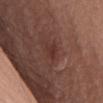Notes:
– follow-up · no biopsy performed (imaged during a skin exam)
– automated lesion analysis · an area of roughly 3.5 mm² and two-axis asymmetry of about 0.35; an average lesion color of about L≈33 a*≈22 b*≈23 (CIELAB), roughly 6 lightness units darker than nearby skin, and a lesion-to-skin contrast of about 6 (normalized; higher = more distinct); an automated nevus-likeness rating near 15 out of 100
– anatomic site · the chest
– subject · male, aged around 55
– diameter · ≈2.5 mm
– image source · ~15 mm crop, total-body skin-cancer survey
– illumination · white-light